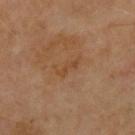Part of a total-body skin-imaging series; this lesion was reviewed on a skin check and was not flagged for biopsy. From the upper back. The subject is a male aged 68 to 72. Cropped from a whole-body photographic skin survey; the tile spans about 15 mm.